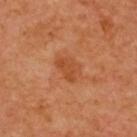workup=total-body-photography surveillance lesion; no biopsy
lesion size=~3.5 mm (longest diameter)
image=~15 mm tile from a whole-body skin photo
site=the upper back
illumination=cross-polarized
patient=female, roughly 40 years of age